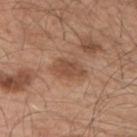No biopsy was performed on this lesion — it was imaged during a full skin examination and was not determined to be concerning. The lesion-visualizer software estimated a footprint of about 6.5 mm², a shape eccentricity near 0.8, and two-axis asymmetry of about 0.25. It also reported a mean CIELAB color near L≈49 a*≈21 b*≈31, about 9 CIELAB-L* units darker than the surrounding skin, and a normalized border contrast of about 7. And it measured a border-irregularity rating of about 3/10, a within-lesion color-variation index near 2/10, and radial color variation of about 0.5. The analysis additionally found an automated nevus-likeness rating near 0 out of 100 and a lesion-detection confidence of about 100/100. Approximately 3.5 mm at its widest. A male subject aged 53 to 57. The tile uses white-light illumination. Located on the left upper arm. A lesion tile, about 15 mm wide, cut from a 3D total-body photograph.